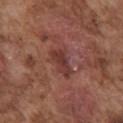follow-up — total-body-photography surveillance lesion; no biopsy | image — ~15 mm tile from a whole-body skin photo | subject — male, approximately 75 years of age | site — the chest.